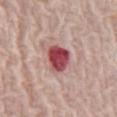Case summary:
* acquisition · 15 mm crop, total-body photography
* subject · female, aged around 65
* body site · the abdomen